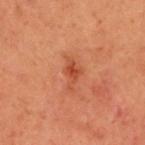Notes:
* biopsy status — catalogued during a skin exam; not biopsied
* site — the head or neck
* illumination — cross-polarized illumination
* image — ~15 mm tile from a whole-body skin photo
* automated metrics — an area of roughly 4.5 mm², an eccentricity of roughly 0.8, and a symmetry-axis asymmetry near 0.4; a mean CIELAB color near L≈41 a*≈27 b*≈32 and a lesion-to-skin contrast of about 6 (normalized; higher = more distinct); an automated nevus-likeness rating near 20 out of 100
* lesion diameter — ~3.5 mm (longest diameter)
* subject — male, about 55 years old